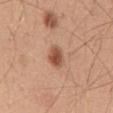biopsy status = imaged on a skin check; not biopsied
size = ~3 mm (longest diameter)
TBP lesion metrics = a lesion color around L≈52 a*≈24 b*≈32 in CIELAB and about 13 CIELAB-L* units darker than the surrounding skin
image source = 15 mm crop, total-body photography
body site = the abdomen
patient = male, aged around 50
illumination = white-light illumination